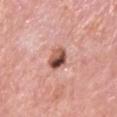Clinical impression:
The lesion was tiled from a total-body skin photograph and was not biopsied.
Acquisition and patient details:
Located on the head or neck. A male subject, roughly 85 years of age or older. The lesion's longest dimension is about 3 mm. Imaged with white-light lighting. A 15 mm close-up extracted from a 3D total-body photography capture.